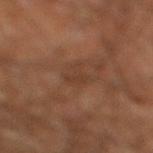workup: catalogued during a skin exam; not biopsied
tile lighting: cross-polarized
size: about 3 mm
subject: male, aged approximately 60
acquisition: 15 mm crop, total-body photography
anatomic site: the right lower leg